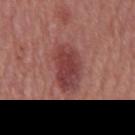Impression: Captured during whole-body skin photography for melanoma surveillance; the lesion was not biopsied. Context: The lesion is located on the mid back. The lesion's longest dimension is about 5.5 mm. A 15 mm close-up extracted from a 3D total-body photography capture. A male patient, approximately 65 years of age.